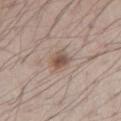The lesion was photographed on a routine skin check and not biopsied; there is no pathology result. The lesion's longest dimension is about 2.5 mm. The subject is a male aged 68–72. The lesion is located on the right upper arm. A roughly 15 mm field-of-view crop from a total-body skin photograph.